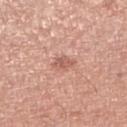  image:
    source: total-body photography crop
    field_of_view_mm: 15
  lesion_size:
    long_diameter_mm_approx: 2.5
  automated_metrics:
    border_irregularity_0_10: 3.0
    peripheral_color_asymmetry: 0.5
    nevus_likeness_0_100: 5
    lesion_detection_confidence_0_100: 100
  site: right lower leg
  patient:
    sex: male
    age_approx: 50
  lighting: white-light